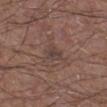follow-up: no biopsy performed (imaged during a skin exam) | illumination: white-light illumination | patient: male, roughly 55 years of age | diameter: about 2.5 mm | image source: ~15 mm tile from a whole-body skin photo | body site: the right lower leg.